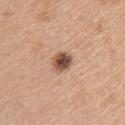Captured during whole-body skin photography for melanoma surveillance; the lesion was not biopsied.
Automated tile analysis of the lesion measured an area of roughly 5.5 mm², an eccentricity of roughly 0.65, and two-axis asymmetry of about 0.15. The software also gave border irregularity of about 1.5 on a 0–10 scale and a peripheral color-asymmetry measure near 2. The software also gave a classifier nevus-likeness of about 95/100 and a lesion-detection confidence of about 100/100.
Cropped from a total-body skin-imaging series; the visible field is about 15 mm.
The subject is a female aged around 45.
Longest diameter approximately 3 mm.
The lesion is located on the right upper arm.